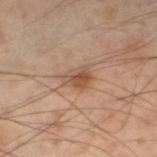Assessment: Captured during whole-body skin photography for melanoma surveillance; the lesion was not biopsied.